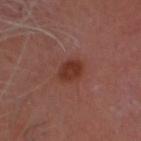site: head or neck
patient:
  sex: male
  age_approx: 60
lighting: cross-polarized
lesion_size:
  long_diameter_mm_approx: 3.5
image:
  source: total-body photography crop
  field_of_view_mm: 15